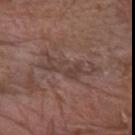Notes:
– follow-up · total-body-photography surveillance lesion; no biopsy
– tile lighting · white-light illumination
– subject · male, aged 78–82
– lesion size · ≈6.5 mm
– image · total-body-photography crop, ~15 mm field of view
– image-analysis metrics · a lesion area of about 9.5 mm² and two-axis asymmetry of about 0.55
– anatomic site · the left forearm